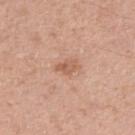This lesion was catalogued during total-body skin photography and was not selected for biopsy.